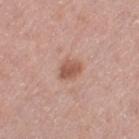The lesion was photographed on a routine skin check and not biopsied; there is no pathology result. The subject is a female aged around 40. This is a white-light tile. A roughly 15 mm field-of-view crop from a total-body skin photograph. The lesion's longest dimension is about 2.5 mm. From the left thigh.